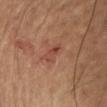The lesion was tiled from a total-body skin photograph and was not biopsied.